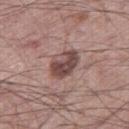Assessment: Recorded during total-body skin imaging; not selected for excision or biopsy. Acquisition and patient details: Located on the right thigh. The total-body-photography lesion software estimated a lesion-detection confidence of about 100/100. Longest diameter approximately 4 mm. A male patient about 60 years old. Imaged with white-light lighting. A roughly 15 mm field-of-view crop from a total-body skin photograph.